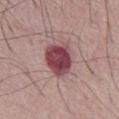Notes:
* body site: the abdomen
* automated lesion analysis: an average lesion color of about L≈44 a*≈27 b*≈16 (CIELAB), about 17 CIELAB-L* units darker than the surrounding skin, and a normalized border contrast of about 12.5; a border-irregularity index near 2/10, a within-lesion color-variation index near 4.5/10, and a peripheral color-asymmetry measure near 1.5
* tile lighting: white-light illumination
* image source: total-body-photography crop, ~15 mm field of view
* lesion diameter: ≈4 mm
* subject: male, in their mid-60s